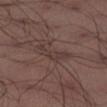{"biopsy_status": "not biopsied; imaged during a skin examination", "lighting": "white-light", "lesion_size": {"long_diameter_mm_approx": 4.5}, "patient": {"sex": "male", "age_approx": 40}, "automated_metrics": {"cielab_L": 36, "cielab_a": 15, "cielab_b": 18, "vs_skin_darker_L": 6.0}, "site": "right lower leg", "image": {"source": "total-body photography crop", "field_of_view_mm": 15}}